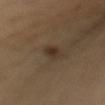workup=imaged on a skin check; not biopsied | subject=female, in their mid-30s | image-analysis metrics=an area of roughly 4 mm², an eccentricity of roughly 0.85, and two-axis asymmetry of about 0.25; a mean CIELAB color near L≈32 a*≈13 b*≈24 and roughly 8 lightness units darker than nearby skin; a border-irregularity rating of about 2.5/10, a color-variation rating of about 3.5/10, and radial color variation of about 1; a lesion-detection confidence of about 100/100 | size=~3 mm (longest diameter) | image=total-body-photography crop, ~15 mm field of view | site=the mid back.